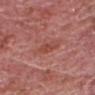Captured during whole-body skin photography for melanoma surveillance; the lesion was not biopsied.
A male patient, aged around 75.
The recorded lesion diameter is about 2.5 mm.
The tile uses white-light illumination.
An algorithmic analysis of the crop reported an automated nevus-likeness rating near 10 out of 100 and a lesion-detection confidence of about 100/100.
Located on the head or neck.
A roughly 15 mm field-of-view crop from a total-body skin photograph.